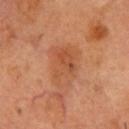follow-up — catalogued during a skin exam; not biopsied | automated metrics — an area of roughly 13 mm², a shape eccentricity near 0.7, and two-axis asymmetry of about 0.25; a lesion color around L≈52 a*≈26 b*≈37 in CIELAB and a normalized lesion–skin contrast near 5.5; a nevus-likeness score of about 10/100 and lesion-presence confidence of about 100/100 | body site — the chest | imaging modality — ~15 mm crop, total-body skin-cancer survey | subject — male, aged approximately 50.